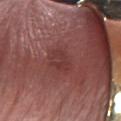Assessment: No biopsy was performed on this lesion — it was imaged during a full skin examination and was not determined to be concerning. Context: The lesion is located on the right forearm. The total-body-photography lesion software estimated border irregularity of about 3 on a 0–10 scale, internal color variation of about 3 on a 0–10 scale, and a peripheral color-asymmetry measure near 1. The subject is a male in their 70s. Cropped from a total-body skin-imaging series; the visible field is about 15 mm. Approximately 2.5 mm at its widest.